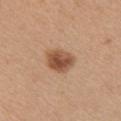Clinical impression: Recorded during total-body skin imaging; not selected for excision or biopsy. Acquisition and patient details: Approximately 3.5 mm at its widest. From the arm. Imaged with white-light lighting. A female patient aged 43 to 47. Cropped from a total-body skin-imaging series; the visible field is about 15 mm. The total-body-photography lesion software estimated an automated nevus-likeness rating near 100 out of 100 and lesion-presence confidence of about 100/100.